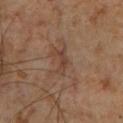<lesion>
  <biopsy_status>not biopsied; imaged during a skin examination</biopsy_status>
  <automated_metrics>
    <border_irregularity_0_10>6.0</border_irregularity_0_10>
    <color_variation_0_10>0.0</color_variation_0_10>
    <nevus_likeness_0_100>0</nevus_likeness_0_100>
    <lesion_detection_confidence_0_100>95</lesion_detection_confidence_0_100>
  </automated_metrics>
  <image>
    <source>total-body photography crop</source>
    <field_of_view_mm>15</field_of_view_mm>
  </image>
  <patient>
    <sex>male</sex>
    <age_approx>60</age_approx>
  </patient>
  <lighting>cross-polarized</lighting>
  <site>left lower leg</site>
</lesion>